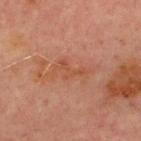biopsy status = no biopsy performed (imaged during a skin exam) | acquisition = total-body-photography crop, ~15 mm field of view | subject = male, aged approximately 70 | body site = the chest | lesion diameter = about 3.5 mm | lighting = cross-polarized illumination.